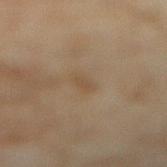Notes:
– follow-up · total-body-photography surveillance lesion; no biopsy
– image · ~15 mm tile from a whole-body skin photo
– subject · female, aged around 60
– anatomic site · the leg
– lesion diameter · about 2.5 mm
– automated metrics · an area of roughly 3.5 mm², an outline eccentricity of about 0.85 (0 = round, 1 = elongated), and a symmetry-axis asymmetry near 0.25; a lesion color around L≈45 a*≈12 b*≈28 in CIELAB, roughly 5 lightness units darker than nearby skin, and a lesion-to-skin contrast of about 4.5 (normalized; higher = more distinct)